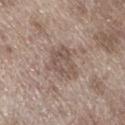Impression: Imaged during a routine full-body skin examination; the lesion was not biopsied and no histopathology is available. Clinical summary: A 15 mm close-up tile from a total-body photography series done for melanoma screening. A male subject, approximately 70 years of age. The total-body-photography lesion software estimated a footprint of about 9 mm², a shape eccentricity near 0.5, and two-axis asymmetry of about 0.35. It also reported an automated nevus-likeness rating near 0 out of 100 and a detector confidence of about 85 out of 100 that the crop contains a lesion. On the left lower leg. Imaged with white-light lighting.